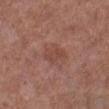lighting: white-light
lesion_size:
  long_diameter_mm_approx: 3.5
site: left lower leg
patient:
  sex: female
  age_approx: 50
automated_metrics:
  cielab_L: 46
  cielab_a: 23
  cielab_b: 26
  vs_skin_darker_L: 6.0
  vs_skin_contrast_norm: 5.5
  border_irregularity_0_10: 3.0
  color_variation_0_10: 2.0
  peripheral_color_asymmetry: 0.5
image:
  source: total-body photography crop
  field_of_view_mm: 15